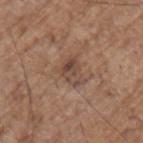<lesion>
  <biopsy_status>not biopsied; imaged during a skin examination</biopsy_status>
  <image>
    <source>total-body photography crop</source>
    <field_of_view_mm>15</field_of_view_mm>
  </image>
  <automated_metrics>
    <cielab_L>45</cielab_L>
    <cielab_a>17</cielab_a>
    <cielab_b>26</cielab_b>
    <vs_skin_darker_L>8.0</vs_skin_darker_L>
    <lesion_detection_confidence_0_100>100</lesion_detection_confidence_0_100>
  </automated_metrics>
  <patient>
    <sex>male</sex>
    <age_approx>70</age_approx>
  </patient>
  <site>left upper arm</site>
</lesion>